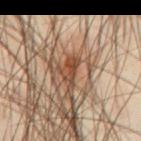Assessment: Part of a total-body skin-imaging series; this lesion was reviewed on a skin check and was not flagged for biopsy. Clinical summary: From the abdomen. A roughly 15 mm field-of-view crop from a total-body skin photograph. The patient is a male about 45 years old.